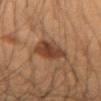Background:
Measured at roughly 4.5 mm in maximum diameter. The lesion is located on the left forearm. A 15 mm close-up tile from a total-body photography series done for melanoma screening. Imaged with cross-polarized lighting. The subject is a male in their mid- to late 30s.